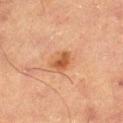Recorded during total-body skin imaging; not selected for excision or biopsy.